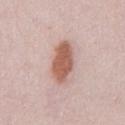biopsy status=no biopsy performed (imaged during a skin exam)
automated lesion analysis=an area of roughly 11 mm² and an eccentricity of roughly 0.85; border irregularity of about 2 on a 0–10 scale and radial color variation of about 1; an automated nevus-likeness rating near 100 out of 100
anatomic site=the chest
image source=15 mm crop, total-body photography
tile lighting=white-light
lesion size=~5 mm (longest diameter)
patient=male, approximately 25 years of age